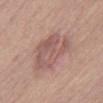<case>
<biopsy_status>not biopsied; imaged during a skin examination</biopsy_status>
<automated_metrics>
  <area_mm2_approx>20.0</area_mm2_approx>
  <eccentricity>0.7</eccentricity>
  <shape_asymmetry>0.3</shape_asymmetry>
  <cielab_L>56</cielab_L>
  <cielab_a>20</cielab_a>
  <cielab_b>23</cielab_b>
  <vs_skin_darker_L>8.0</vs_skin_darker_L>
  <vs_skin_contrast_norm>6.0</vs_skin_contrast_norm>
</automated_metrics>
<site>abdomen</site>
<lighting>white-light</lighting>
<image>
  <source>total-body photography crop</source>
  <field_of_view_mm>15</field_of_view_mm>
</image>
<patient>
  <sex>male</sex>
  <age_approx>60</age_approx>
</patient>
</case>